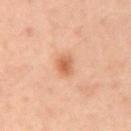Clinical impression:
No biopsy was performed on this lesion — it was imaged during a full skin examination and was not determined to be concerning.
Image and clinical context:
A close-up tile cropped from a whole-body skin photograph, about 15 mm across. Imaged with cross-polarized lighting. The lesion is on the abdomen. A male patient approximately 60 years of age. Measured at roughly 2.5 mm in maximum diameter.